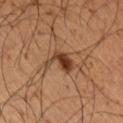No biopsy was performed on this lesion — it was imaged during a full skin examination and was not determined to be concerning. The subject is a male aged around 50. A 15 mm close-up extracted from a 3D total-body photography capture. From the upper back.